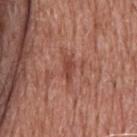<record>
  <biopsy_status>not biopsied; imaged during a skin examination</biopsy_status>
  <patient>
    <sex>male</sex>
    <age_approx>60</age_approx>
  </patient>
  <site>upper back</site>
  <lesion_size>
    <long_diameter_mm_approx>3.0</long_diameter_mm_approx>
  </lesion_size>
  <automated_metrics>
    <area_mm2_approx>3.0</area_mm2_approx>
    <eccentricity>0.9</eccentricity>
    <vs_skin_darker_L>9.0</vs_skin_darker_L>
    <vs_skin_contrast_norm>7.0</vs_skin_contrast_norm>
    <nevus_likeness_0_100>0</nevus_likeness_0_100>
    <lesion_detection_confidence_0_100>80</lesion_detection_confidence_0_100>
  </automated_metrics>
  <lighting>white-light</lighting>
  <image>
    <source>total-body photography crop</source>
    <field_of_view_mm>15</field_of_view_mm>
  </image>
</record>